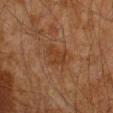notes: catalogued during a skin exam; not biopsied | subject: male, aged around 45 | image: 15 mm crop, total-body photography | automated metrics: an area of roughly 4.5 mm² and a shape eccentricity near 0.8; a lesion–skin lightness drop of about 5 and a normalized lesion–skin contrast near 6; internal color variation of about 1 on a 0–10 scale and radial color variation of about 0 | body site: the right forearm.